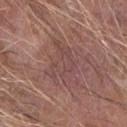Assessment:
Imaged during a routine full-body skin examination; the lesion was not biopsied and no histopathology is available.
Image and clinical context:
A close-up tile cropped from a whole-body skin photograph, about 15 mm across. A male patient roughly 65 years of age. From the arm.